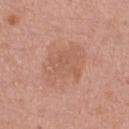Impression: The lesion was photographed on a routine skin check and not biopsied; there is no pathology result. Clinical summary: The lesion is located on the right thigh. A 15 mm close-up extracted from a 3D total-body photography capture. A female patient, about 55 years old.